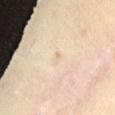{"image": {"source": "total-body photography crop", "field_of_view_mm": 15}, "patient": {"sex": "female", "age_approx": 65}, "site": "front of the torso", "lighting": "cross-polarized"}